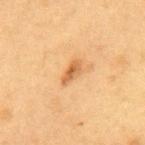Case summary:
– biopsy status · imaged on a skin check; not biopsied